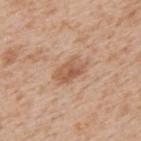Q: Was a biopsy performed?
A: catalogued during a skin exam; not biopsied
Q: Patient demographics?
A: male, aged 63–67
Q: What kind of image is this?
A: 15 mm crop, total-body photography
Q: Where on the body is the lesion?
A: the back